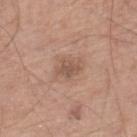Findings:
• notes — catalogued during a skin exam; not biopsied
• lesion diameter — about 2.5 mm
• patient — male, in their mid-60s
• location — the right lower leg
• lighting — white-light illumination
• imaging modality — ~15 mm crop, total-body skin-cancer survey